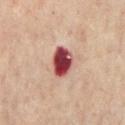Q: Was this lesion biopsied?
A: catalogued during a skin exam; not biopsied
Q: Lesion size?
A: about 3.5 mm
Q: Patient demographics?
A: male, aged around 65
Q: What is the imaging modality?
A: ~15 mm tile from a whole-body skin photo
Q: What lighting was used for the tile?
A: cross-polarized
Q: Lesion location?
A: the front of the torso
Q: Automated lesion metrics?
A: roughly 23 lightness units darker than nearby skin and a normalized border contrast of about 15.5; a nevus-likeness score of about 0/100 and lesion-presence confidence of about 100/100